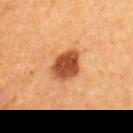image: 15 mm crop, total-body photography
body site: the left upper arm
subject: female, roughly 55 years of age